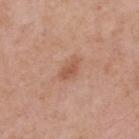Findings:
• subject — male, in their mid- to late 50s
• automated metrics — a lesion area of about 4 mm², an outline eccentricity of about 0.85 (0 = round, 1 = elongated), and two-axis asymmetry of about 0.25; a lesion color around L≈55 a*≈23 b*≈31 in CIELAB, roughly 8 lightness units darker than nearby skin, and a lesion-to-skin contrast of about 6 (normalized; higher = more distinct); a within-lesion color-variation index near 1/10 and a peripheral color-asymmetry measure near 0.5
• anatomic site — the upper back
• lesion diameter — about 3 mm
• imaging modality — ~15 mm crop, total-body skin-cancer survey